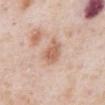  biopsy_status: not biopsied; imaged during a skin examination
  image:
    source: total-body photography crop
    field_of_view_mm: 15
  lighting: white-light
  site: abdomen
  patient:
    sex: male
    age_approx: 80
  lesion_size:
    long_diameter_mm_approx: 3.5